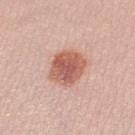workup: imaged on a skin check; not biopsied
anatomic site: the leg
image source: ~15 mm crop, total-body skin-cancer survey
patient: female, about 25 years old